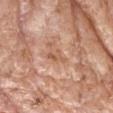workup: catalogued during a skin exam; not biopsied
body site: the head or neck
subject: male, in their mid- to late 70s
imaging modality: ~15 mm tile from a whole-body skin photo
tile lighting: white-light illumination